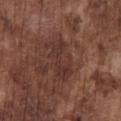{
  "patient": {
    "sex": "male",
    "age_approx": 75
  },
  "site": "chest",
  "lesion_size": {
    "long_diameter_mm_approx": 5.0
  },
  "automated_metrics": {
    "vs_skin_darker_L": 7.0,
    "vs_skin_contrast_norm": 7.0,
    "color_variation_0_10": 3.0,
    "peripheral_color_asymmetry": 1.0
  },
  "lighting": "white-light",
  "image": {
    "source": "total-body photography crop",
    "field_of_view_mm": 15
  }
}